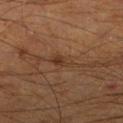Findings:
– biopsy status — catalogued during a skin exam; not biopsied
– patient — male, approximately 65 years of age
– lesion diameter — about 3 mm
– body site — the right lower leg
– image-analysis metrics — roughly 6 lightness units darker than nearby skin and a normalized border contrast of about 6; a classifier nevus-likeness of about 5/100 and a detector confidence of about 100 out of 100 that the crop contains a lesion
– illumination — cross-polarized illumination
– image source — ~15 mm tile from a whole-body skin photo